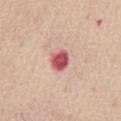notes: imaged on a skin check; not biopsied
patient: male, approximately 60 years of age
image-analysis metrics: an eccentricity of roughly 0.55; a mean CIELAB color near L≈56 a*≈33 b*≈24, roughly 18 lightness units darker than nearby skin, and a lesion-to-skin contrast of about 11 (normalized; higher = more distinct); a classifier nevus-likeness of about 0/100 and a detector confidence of about 100 out of 100 that the crop contains a lesion
illumination: white-light
body site: the abdomen
image source: 15 mm crop, total-body photography